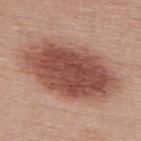Q: Is there a histopathology result?
A: catalogued during a skin exam; not biopsied
Q: Lesion location?
A: the upper back
Q: What are the patient's age and sex?
A: female, in their 50s
Q: What is the imaging modality?
A: ~15 mm tile from a whole-body skin photo